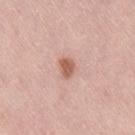Assessment: No biopsy was performed on this lesion — it was imaged during a full skin examination and was not determined to be concerning. Clinical summary: The lesion is located on the lower back. A roughly 15 mm field-of-view crop from a total-body skin photograph. Automated tile analysis of the lesion measured a lesion area of about 3.5 mm², an eccentricity of roughly 0.7, and a symmetry-axis asymmetry near 0.25. The software also gave a within-lesion color-variation index near 1.5/10 and peripheral color asymmetry of about 0.5. This is a white-light tile. A female subject, aged 58–62. Longest diameter approximately 2.5 mm.